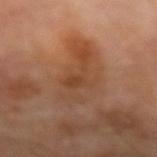Clinical impression: Recorded during total-body skin imaging; not selected for excision or biopsy. Context: A male patient aged 68–72. The total-body-photography lesion software estimated a footprint of about 14 mm², an outline eccentricity of about 0.9 (0 = round, 1 = elongated), and a shape-asymmetry score of about 0.45 (0 = symmetric). The software also gave an average lesion color of about L≈43 a*≈21 b*≈32 (CIELAB) and a lesion-to-skin contrast of about 6 (normalized; higher = more distinct). Longest diameter approximately 7 mm. On the left forearm. Cropped from a total-body skin-imaging series; the visible field is about 15 mm. This is a cross-polarized tile.